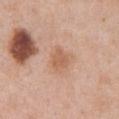Q: Is there a histopathology result?
A: imaged on a skin check; not biopsied
Q: What lighting was used for the tile?
A: white-light illumination
Q: Patient demographics?
A: male, in their 70s
Q: Lesion location?
A: the abdomen
Q: What is the imaging modality?
A: ~15 mm tile from a whole-body skin photo
Q: Automated lesion metrics?
A: a footprint of about 6 mm², an eccentricity of roughly 0.65, and a shape-asymmetry score of about 0.2 (0 = symmetric)